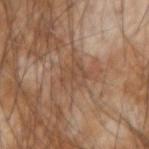The lesion was tiled from a total-body skin photograph and was not biopsied. A male subject, aged 53–57. Cropped from a whole-body photographic skin survey; the tile spans about 15 mm. Located on the right forearm. The total-body-photography lesion software estimated a lesion area of about 7 mm², an eccentricity of roughly 0.5, and a symmetry-axis asymmetry near 0.25. The software also gave border irregularity of about 3.5 on a 0–10 scale, internal color variation of about 2.5 on a 0–10 scale, and radial color variation of about 1. The software also gave a classifier nevus-likeness of about 0/100. Longest diameter approximately 3.5 mm. This is a cross-polarized tile.